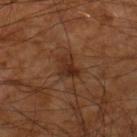follow-up — catalogued during a skin exam; not biopsied | body site — the right lower leg | diameter — ≈3 mm | imaging modality — ~15 mm tile from a whole-body skin photo | lighting — cross-polarized | patient — male, in their 60s.